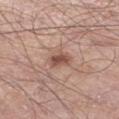| field | value |
|---|---|
| image source | 15 mm crop, total-body photography |
| illumination | white-light |
| patient | male, in their 70s |
| size | ≈2.5 mm |
| site | the leg |